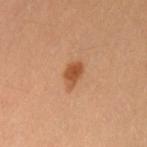workup = imaged on a skin check; not biopsied | imaging modality = 15 mm crop, total-body photography | anatomic site = the left upper arm | TBP lesion metrics = a footprint of about 4.5 mm², an eccentricity of roughly 0.75, and two-axis asymmetry of about 0.2; a lesion color around L≈52 a*≈25 b*≈38 in CIELAB and roughly 12 lightness units darker than nearby skin; a nevus-likeness score of about 95/100 and a detector confidence of about 100 out of 100 that the crop contains a lesion | illumination = cross-polarized | subject = female, in their mid-30s.